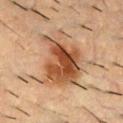Clinical summary: The subject is a male aged approximately 55. Located on the back. A roughly 15 mm field-of-view crop from a total-body skin photograph.